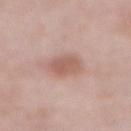From the front of the torso. The patient is a male aged approximately 55. A roughly 15 mm field-of-view crop from a total-body skin photograph. About 3 mm across.